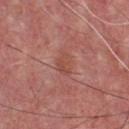Imaged during a routine full-body skin examination; the lesion was not biopsied and no histopathology is available.
On the chest.
Automated image analysis of the tile measured an automated nevus-likeness rating near 0 out of 100 and lesion-presence confidence of about 100/100.
Captured under white-light illumination.
A male subject, aged approximately 55.
Cropped from a whole-body photographic skin survey; the tile spans about 15 mm.